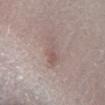Imaged during a routine full-body skin examination; the lesion was not biopsied and no histopathology is available.
On the right lower leg.
A roughly 15 mm field-of-view crop from a total-body skin photograph.
The subject is a male aged 78–82.
This is a white-light tile.